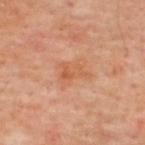Assessment:
The lesion was tiled from a total-body skin photograph and was not biopsied.
Acquisition and patient details:
A close-up tile cropped from a whole-body skin photograph, about 15 mm across. From the upper back. The patient is a male in their mid-60s.